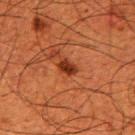| field | value |
|---|---|
| follow-up | imaged on a skin check; not biopsied |
| imaging modality | total-body-photography crop, ~15 mm field of view |
| location | the right upper arm |
| subject | male, in their 50s |
| size | ≈2.5 mm |
| lighting | cross-polarized illumination |